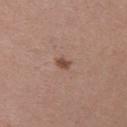The lesion was photographed on a routine skin check and not biopsied; there is no pathology result.
A female patient, aged around 30.
From the left upper arm.
A roughly 15 mm field-of-view crop from a total-body skin photograph.
About 2 mm across.
Automated tile analysis of the lesion measured an area of roughly 2.5 mm², a shape eccentricity near 0.7, and a shape-asymmetry score of about 0.25 (0 = symmetric). It also reported an average lesion color of about L≈48 a*≈20 b*≈27 (CIELAB) and roughly 10 lightness units darker than nearby skin. The analysis additionally found a nevus-likeness score of about 75/100 and a lesion-detection confidence of about 100/100.
Captured under white-light illumination.